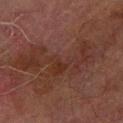| field | value |
|---|---|
| workup | total-body-photography surveillance lesion; no biopsy |
| image | total-body-photography crop, ~15 mm field of view |
| lesion diameter | about 13 mm |
| patient | male, aged around 75 |
| TBP lesion metrics | an average lesion color of about L≈26 a*≈17 b*≈21 (CIELAB) and a lesion-to-skin contrast of about 6 (normalized; higher = more distinct) |
| body site | the right forearm |